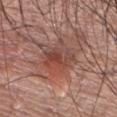Notes:
• follow-up: no biopsy performed (imaged during a skin exam)
• site: the chest
• subject: male, about 60 years old
• image: total-body-photography crop, ~15 mm field of view